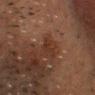Imaged during a routine full-body skin examination; the lesion was not biopsied and no histopathology is available. About 3 mm across. This image is a 15 mm lesion crop taken from a total-body photograph. The total-body-photography lesion software estimated an area of roughly 3 mm² and two-axis asymmetry of about 0.35. It also reported a mean CIELAB color near L≈24 a*≈17 b*≈22, a lesion–skin lightness drop of about 5, and a lesion-to-skin contrast of about 5.5 (normalized; higher = more distinct). Imaged with cross-polarized lighting. A male subject, about 50 years old. On the head or neck.